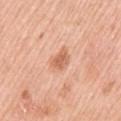• follow-up · no biopsy performed (imaged during a skin exam)
• lighting · white-light illumination
• subject · male, in their mid-50s
• body site · the arm
• imaging modality · total-body-photography crop, ~15 mm field of view
• automated metrics · a lesion-to-skin contrast of about 7 (normalized; higher = more distinct); a classifier nevus-likeness of about 75/100 and a lesion-detection confidence of about 100/100